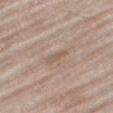This lesion was catalogued during total-body skin photography and was not selected for biopsy. A lesion tile, about 15 mm wide, cut from a 3D total-body photograph. The lesion is on the right thigh. The recorded lesion diameter is about 3 mm. The subject is a male roughly 80 years of age.